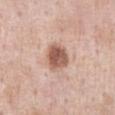The lesion was tiled from a total-body skin photograph and was not biopsied. A male subject, roughly 55 years of age. The lesion-visualizer software estimated an average lesion color of about L≈57 a*≈21 b*≈28 (CIELAB) and a normalized lesion–skin contrast near 10. The analysis additionally found a border-irregularity index near 2/10 and radial color variation of about 1.5. Located on the chest. A 15 mm crop from a total-body photograph taken for skin-cancer surveillance.